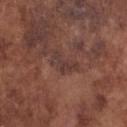| key | value |
|---|---|
| anatomic site | the chest |
| subject | male, aged 73–77 |
| image | ~15 mm crop, total-body skin-cancer survey |
| illumination | white-light |
| TBP lesion metrics | about 6 CIELAB-L* units darker than the surrounding skin and a normalized lesion–skin contrast near 6; a nevus-likeness score of about 0/100 and a lesion-detection confidence of about 65/100 |
| diameter | ~4 mm (longest diameter) |